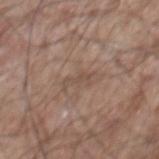biopsy status: no biopsy performed (imaged during a skin exam)
patient: male, aged 53 to 57
automated metrics: a lesion area of about 3 mm², an outline eccentricity of about 0.9 (0 = round, 1 = elongated), and two-axis asymmetry of about 0.45; a border-irregularity index near 7.5/10, internal color variation of about 0 on a 0–10 scale, and peripheral color asymmetry of about 0
body site: the mid back
image: ~15 mm crop, total-body skin-cancer survey